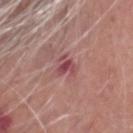Findings:
- anatomic site — the head or neck
- lesion diameter — ~3 mm (longest diameter)
- image source — total-body-photography crop, ~15 mm field of view
- illumination — white-light
- patient — male, aged around 65